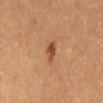Impression: No biopsy was performed on this lesion — it was imaged during a full skin examination and was not determined to be concerning. Image and clinical context: A male subject, about 60 years old. A lesion tile, about 15 mm wide, cut from a 3D total-body photograph. The lesion is located on the abdomen.